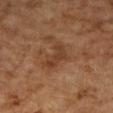{"biopsy_status": "not biopsied; imaged during a skin examination", "patient": {"sex": "female", "age_approx": 60}, "image": {"source": "total-body photography crop", "field_of_view_mm": 15}, "site": "arm", "automated_metrics": {"area_mm2_approx": 7.5, "shape_asymmetry": 0.45, "cielab_L": 34, "cielab_a": 18, "cielab_b": 28, "vs_skin_darker_L": 7.0, "vs_skin_contrast_norm": 6.5}, "lesion_size": {"long_diameter_mm_approx": 4.0}, "lighting": "cross-polarized"}